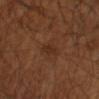| field | value |
|---|---|
| image source | total-body-photography crop, ~15 mm field of view |
| subject | male, aged approximately 65 |